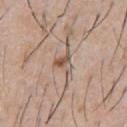Clinical impression: The lesion was tiled from a total-body skin photograph and was not biopsied. Background: Captured under white-light illumination. A roughly 15 mm field-of-view crop from a total-body skin photograph. A male patient, aged approximately 60. The lesion is located on the chest. Approximately 3 mm at its widest.